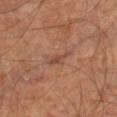No biopsy was performed on this lesion — it was imaged during a full skin examination and was not determined to be concerning.
The patient is a male aged around 65.
The lesion's longest dimension is about 3.5 mm.
From the leg.
This is a cross-polarized tile.
Cropped from a whole-body photographic skin survey; the tile spans about 15 mm.
Automated image analysis of the tile measured lesion-presence confidence of about 65/100.